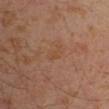The lesion was photographed on a routine skin check and not biopsied; there is no pathology result. The subject is a male aged around 30. An algorithmic analysis of the crop reported an average lesion color of about L≈45 a*≈18 b*≈32 (CIELAB) and a lesion-to-skin contrast of about 5 (normalized; higher = more distinct). The recorded lesion diameter is about 2.5 mm. The lesion is located on the left arm. Imaged with cross-polarized lighting. A 15 mm close-up tile from a total-body photography series done for melanoma screening.